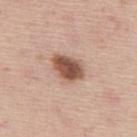The lesion was photographed on a routine skin check and not biopsied; there is no pathology result.
Approximately 4 mm at its widest.
The tile uses white-light illumination.
From the back.
Automated image analysis of the tile measured an average lesion color of about L≈52 a*≈21 b*≈28 (CIELAB), a lesion–skin lightness drop of about 17, and a lesion-to-skin contrast of about 11 (normalized; higher = more distinct). The analysis additionally found a border-irregularity rating of about 2/10, internal color variation of about 4.5 on a 0–10 scale, and peripheral color asymmetry of about 1.5.
A lesion tile, about 15 mm wide, cut from a 3D total-body photograph.
A male subject approximately 45 years of age.